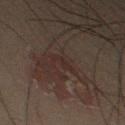Part of a total-body skin-imaging series; this lesion was reviewed on a skin check and was not flagged for biopsy.
A male patient about 65 years old.
From the left thigh.
A roughly 15 mm field-of-view crop from a total-body skin photograph.